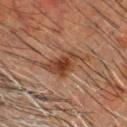The subject is a male about 50 years old.
A lesion tile, about 15 mm wide, cut from a 3D total-body photograph.
Imaged with cross-polarized lighting.
Longest diameter approximately 6 mm.
Automated image analysis of the tile measured a border-irregularity index near 5/10 and a color-variation rating of about 4.5/10.
Located on the head or neck.